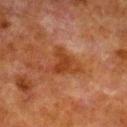Captured during whole-body skin photography for melanoma surveillance; the lesion was not biopsied.